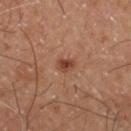<tbp_lesion>
  <patient>
    <sex>male</sex>
    <age_approx>60</age_approx>
  </patient>
  <lesion_size>
    <long_diameter_mm_approx>2.0</long_diameter_mm_approx>
  </lesion_size>
  <site>leg</site>
  <image>
    <source>total-body photography crop</source>
    <field_of_view_mm>15</field_of_view_mm>
  </image>
  <automated_metrics>
    <cielab_L>38</cielab_L>
    <cielab_a>22</cielab_a>
    <cielab_b>28</cielab_b>
    <vs_skin_darker_L>10.0</vs_skin_darker_L>
    <nevus_likeness_0_100>85</nevus_likeness_0_100>
    <lesion_detection_confidence_0_100>100</lesion_detection_confidence_0_100>
  </automated_metrics>
</tbp_lesion>